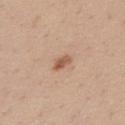A region of skin cropped from a whole-body photographic capture, roughly 15 mm wide.
The patient is a male aged around 40.
Longest diameter approximately 2.5 mm.
From the chest.
Imaged with white-light lighting.
The total-body-photography lesion software estimated internal color variation of about 3 on a 0–10 scale. The software also gave a nevus-likeness score of about 80/100 and lesion-presence confidence of about 100/100.